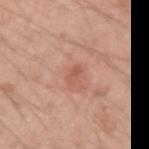biopsy_status: not biopsied; imaged during a skin examination
patient:
  sex: male
  age_approx: 20
lighting: white-light
site: left upper arm
image:
  source: total-body photography crop
  field_of_view_mm: 15
lesion_size:
  long_diameter_mm_approx: 2.5